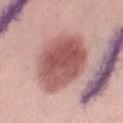Notes:
* body site · the right thigh
* subject · female, aged 63–67
* acquisition · ~15 mm tile from a whole-body skin photo